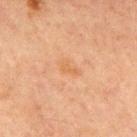biopsy_status: not biopsied; imaged during a skin examination
patient:
  sex: male
  age_approx: 65
site: chest
image:
  source: total-body photography crop
  field_of_view_mm: 15
lighting: cross-polarized